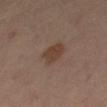Recorded during total-body skin imaging; not selected for excision or biopsy.
This image is a 15 mm lesion crop taken from a total-body photograph.
A female subject, aged 43–47.
Measured at roughly 3.5 mm in maximum diameter.
Located on the right thigh.